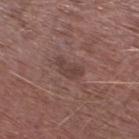biopsy status — total-body-photography surveillance lesion; no biopsy
automated lesion analysis — a footprint of about 5 mm² and a shape eccentricity near 0.75; a border-irregularity index near 3.5/10, a within-lesion color-variation index near 2/10, and peripheral color asymmetry of about 0.5
lighting — white-light
imaging modality — 15 mm crop, total-body photography
lesion size — ≈3 mm
subject — male, aged 73 to 77
body site — the left lower leg